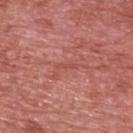The lesion was tiled from a total-body skin photograph and was not biopsied. A male patient approximately 70 years of age. A 15 mm close-up extracted from a 3D total-body photography capture. The total-body-photography lesion software estimated a footprint of about 1.5 mm² and a symmetry-axis asymmetry near 0.3. It also reported a lesion color around L≈51 a*≈30 b*≈29 in CIELAB, roughly 6 lightness units darker than nearby skin, and a normalized lesion–skin contrast near 4.5. This is a white-light tile. The lesion's longest dimension is about 2.5 mm. The lesion is on the upper back.